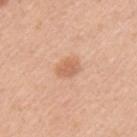Image and clinical context:
The recorded lesion diameter is about 2.5 mm. The lesion is on the arm. Imaged with white-light lighting. A 15 mm crop from a total-body photograph taken for skin-cancer surveillance. A female subject, aged 28 to 32.